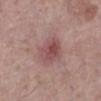Assessment: No biopsy was performed on this lesion — it was imaged during a full skin examination and was not determined to be concerning. Context: Captured under white-light illumination. A female patient, in their mid-60s. The recorded lesion diameter is about 3.5 mm. On the leg. A region of skin cropped from a whole-body photographic capture, roughly 15 mm wide.